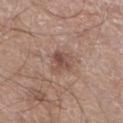The lesion was photographed on a routine skin check and not biopsied; there is no pathology result.